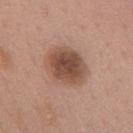Clinical summary:
From the mid back. A 15 mm close-up tile from a total-body photography series done for melanoma screening. A female patient aged around 50.